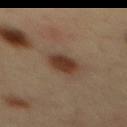Case summary:
* biopsy status · total-body-photography surveillance lesion; no biopsy
* patient · male, approximately 35 years of age
* anatomic site · the mid back
* TBP lesion metrics · a lesion color around L≈32 a*≈17 b*≈25 in CIELAB, roughly 12 lightness units darker than nearby skin, and a normalized lesion–skin contrast near 11; a nevus-likeness score of about 100/100 and a lesion-detection confidence of about 100/100
* lesion size · ≈3 mm
* tile lighting · cross-polarized illumination
* image · total-body-photography crop, ~15 mm field of view